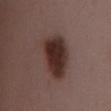notes = no biopsy performed (imaged during a skin exam); acquisition = ~15 mm tile from a whole-body skin photo; patient = female, aged 33–37; body site = the mid back.